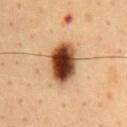notes: no biopsy performed (imaged during a skin exam)
lesion size: ≈5.5 mm
patient: male, in their mid-60s
image source: total-body-photography crop, ~15 mm field of view
anatomic site: the front of the torso
automated lesion analysis: a footprint of about 15 mm², an eccentricity of roughly 0.75, and two-axis asymmetry of about 0.1; border irregularity of about 1 on a 0–10 scale; a nevus-likeness score of about 100/100 and a lesion-detection confidence of about 100/100
illumination: cross-polarized illumination